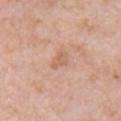Assessment:
This lesion was catalogued during total-body skin photography and was not selected for biopsy.
Background:
The lesion's longest dimension is about 2.5 mm. Cropped from a whole-body photographic skin survey; the tile spans about 15 mm. The lesion is on the chest. This is a white-light tile. An algorithmic analysis of the crop reported a lesion color around L≈62 a*≈21 b*≈31 in CIELAB and a normalized border contrast of about 5. It also reported a classifier nevus-likeness of about 0/100 and a detector confidence of about 100 out of 100 that the crop contains a lesion. A male patient, aged 48 to 52.